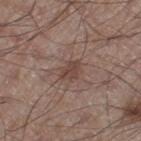workup=catalogued during a skin exam; not biopsied
imaging modality=~15 mm tile from a whole-body skin photo
subject=male, approximately 60 years of age
lesion size=~2.5 mm (longest diameter)
site=the left thigh
lighting=white-light